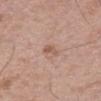workup: imaged on a skin check; not biopsied | patient: male, roughly 50 years of age | image-analysis metrics: an outline eccentricity of about 0.85 (0 = round, 1 = elongated); an average lesion color of about L≈56 a*≈20 b*≈28 (CIELAB), a lesion–skin lightness drop of about 8, and a lesion-to-skin contrast of about 6 (normalized; higher = more distinct); a border-irregularity index near 3.5/10 and a peripheral color-asymmetry measure near 0 | image source: ~15 mm tile from a whole-body skin photo | size: ~2.5 mm (longest diameter) | illumination: white-light illumination | location: the abdomen.